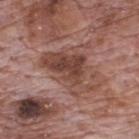| key | value |
|---|---|
| notes | no biopsy performed (imaged during a skin exam) |
| image | ~15 mm tile from a whole-body skin photo |
| anatomic site | the upper back |
| tile lighting | white-light illumination |
| subject | male, aged 68–72 |
| diameter | ≈7 mm |